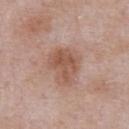Recorded during total-body skin imaging; not selected for excision or biopsy.
The patient is a male roughly 55 years of age.
Approximately 4 mm at its widest.
Automated tile analysis of the lesion measured a footprint of about 10 mm² and two-axis asymmetry of about 0.3. The analysis additionally found an average lesion color of about L≈53 a*≈21 b*≈28 (CIELAB) and a lesion-to-skin contrast of about 7 (normalized; higher = more distinct).
A region of skin cropped from a whole-body photographic capture, roughly 15 mm wide.
Located on the abdomen.
Imaged with white-light lighting.